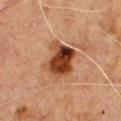| feature | finding |
|---|---|
| biopsy status | total-body-photography surveillance lesion; no biopsy |
| tile lighting | cross-polarized |
| subject | male, aged 48–52 |
| location | the chest |
| TBP lesion metrics | an outline eccentricity of about 0.7 (0 = round, 1 = elongated); a within-lesion color-variation index near 7.5/10 and a peripheral color-asymmetry measure near 2.5; a nevus-likeness score of about 100/100 and a detector confidence of about 100 out of 100 that the crop contains a lesion |
| image | ~15 mm crop, total-body skin-cancer survey |